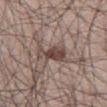* follow-up: no biopsy performed (imaged during a skin exam)
* location: the right thigh
* illumination: white-light illumination
* imaging modality: ~15 mm crop, total-body skin-cancer survey
* subject: male, about 30 years old
* lesion size: about 5 mm
* TBP lesion metrics: a lesion–skin lightness drop of about 12 and a lesion-to-skin contrast of about 9 (normalized; higher = more distinct); a classifier nevus-likeness of about 95/100